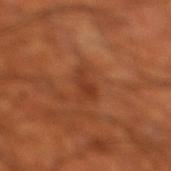Recorded during total-body skin imaging; not selected for excision or biopsy.
Automated image analysis of the tile measured a nevus-likeness score of about 0/100 and a detector confidence of about 100 out of 100 that the crop contains a lesion.
A 15 mm crop from a total-body photograph taken for skin-cancer surveillance.
This is a cross-polarized tile.
On the leg.
A male patient, aged 68–72.